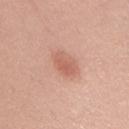follow-up — imaged on a skin check; not biopsied | image source — total-body-photography crop, ~15 mm field of view | body site — the upper back | subject — male, approximately 55 years of age.